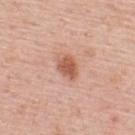{
  "biopsy_status": "not biopsied; imaged during a skin examination",
  "site": "upper back",
  "lesion_size": {
    "long_diameter_mm_approx": 3.0
  },
  "lighting": "white-light",
  "patient": {
    "sex": "male",
    "age_approx": 55
  },
  "image": {
    "source": "total-body photography crop",
    "field_of_view_mm": 15
  }
}